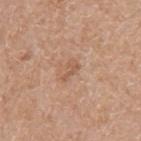biopsy_status: not biopsied; imaged during a skin examination